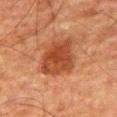Imaged during a routine full-body skin examination; the lesion was not biopsied and no histopathology is available.
Located on the right thigh.
Captured under cross-polarized illumination.
Approximately 5 mm at its widest.
A male subject, approximately 80 years of age.
Automated image analysis of the tile measured an average lesion color of about L≈36 a*≈24 b*≈31 (CIELAB). It also reported border irregularity of about 2 on a 0–10 scale, a color-variation rating of about 3/10, and radial color variation of about 1. And it measured a nevus-likeness score of about 90/100 and a lesion-detection confidence of about 100/100.
A 15 mm crop from a total-body photograph taken for skin-cancer surveillance.